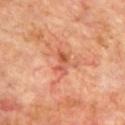Measured at roughly 3 mm in maximum diameter. A 15 mm crop from a total-body photograph taken for skin-cancer surveillance. The lesion is on the chest. Captured under cross-polarized illumination. The subject is a male in their 70s.